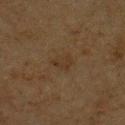notes — no biopsy performed (imaged during a skin exam) | subject — male, aged around 75 | acquisition — ~15 mm tile from a whole-body skin photo | tile lighting — cross-polarized | location — the chest | lesion size — about 2.5 mm.